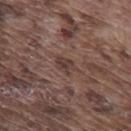biopsy status: imaged on a skin check; not biopsied
body site: the mid back
lighting: white-light
imaging modality: ~15 mm tile from a whole-body skin photo
automated metrics: a lesion area of about 4 mm², an outline eccentricity of about 0.7 (0 = round, 1 = elongated), and a shape-asymmetry score of about 0.35 (0 = symmetric); about 7 CIELAB-L* units darker than the surrounding skin and a normalized border contrast of about 6.5; a border-irregularity rating of about 3.5/10, a color-variation rating of about 2.5/10, and peripheral color asymmetry of about 1; an automated nevus-likeness rating near 0 out of 100 and a lesion-detection confidence of about 80/100
patient: male, approximately 75 years of age
lesion diameter: ~3 mm (longest diameter)